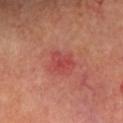Impression:
Recorded during total-body skin imaging; not selected for excision or biopsy.
Context:
A female patient approximately 75 years of age. This is a cross-polarized tile. The recorded lesion diameter is about 3 mm. From the head or neck. Automated image analysis of the tile measured an automated nevus-likeness rating near 0 out of 100 and a detector confidence of about 100 out of 100 that the crop contains a lesion. A lesion tile, about 15 mm wide, cut from a 3D total-body photograph.